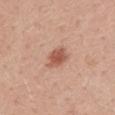No biopsy was performed on this lesion — it was imaged during a full skin examination and was not determined to be concerning.
On the mid back.
This image is a 15 mm lesion crop taken from a total-body photograph.
A male subject approximately 25 years of age.
Automated tile analysis of the lesion measured a lesion color around L≈55 a*≈26 b*≈30 in CIELAB, roughly 12 lightness units darker than nearby skin, and a normalized lesion–skin contrast near 8. The analysis additionally found a border-irregularity rating of about 1/10, internal color variation of about 2.5 on a 0–10 scale, and a peripheral color-asymmetry measure near 1. It also reported an automated nevus-likeness rating near 95 out of 100 and a detector confidence of about 100 out of 100 that the crop contains a lesion.
The recorded lesion diameter is about 2.5 mm.